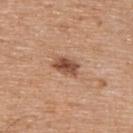Part of a total-body skin-imaging series; this lesion was reviewed on a skin check and was not flagged for biopsy. This is a white-light tile. The total-body-photography lesion software estimated a footprint of about 5.5 mm² and a symmetry-axis asymmetry near 0.2. The analysis additionally found a lesion color around L≈50 a*≈22 b*≈31 in CIELAB and a lesion–skin lightness drop of about 14. The analysis additionally found a lesion-detection confidence of about 100/100. The lesion is on the upper back. Longest diameter approximately 3 mm. A male patient, in their mid- to late 60s. Cropped from a total-body skin-imaging series; the visible field is about 15 mm.